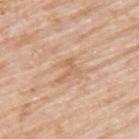tile lighting=white-light
size=≈3 mm
location=the left upper arm
subject=male, in their mid-70s
acquisition=~15 mm tile from a whole-body skin photo
automated metrics=a shape eccentricity near 0.8; an average lesion color of about L≈63 a*≈21 b*≈34 (CIELAB); lesion-presence confidence of about 95/100